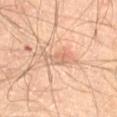Recorded during total-body skin imaging; not selected for excision or biopsy. The lesion is located on the right thigh. A close-up tile cropped from a whole-body skin photograph, about 15 mm across. Imaged with cross-polarized lighting. The lesion-visualizer software estimated a footprint of about 6.5 mm² and an outline eccentricity of about 0.9 (0 = round, 1 = elongated). And it measured a normalized lesion–skin contrast near 5. It also reported a border-irregularity rating of about 4.5/10, a color-variation rating of about 5.5/10, and peripheral color asymmetry of about 2. The patient is a male approximately 65 years of age.